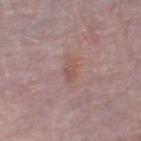workup — catalogued during a skin exam; not biopsied
image — ~15 mm tile from a whole-body skin photo
automated metrics — a lesion area of about 3.5 mm², an outline eccentricity of about 0.85 (0 = round, 1 = elongated), and a symmetry-axis asymmetry near 0.3; an average lesion color of about L≈53 a*≈20 b*≈23 (CIELAB), a lesion–skin lightness drop of about 6, and a lesion-to-skin contrast of about 5.5 (normalized; higher = more distinct)
body site — the right thigh
subject — male, roughly 75 years of age